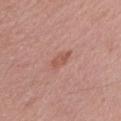Q: Is there a histopathology result?
A: no biopsy performed (imaged during a skin exam)
Q: Patient demographics?
A: female, roughly 50 years of age
Q: What kind of image is this?
A: ~15 mm crop, total-body skin-cancer survey
Q: Illumination type?
A: white-light illumination
Q: What did automated image analysis measure?
A: roughly 8 lightness units darker than nearby skin; border irregularity of about 3 on a 0–10 scale; a classifier nevus-likeness of about 10/100 and a lesion-detection confidence of about 100/100
Q: How large is the lesion?
A: about 3 mm
Q: Lesion location?
A: the left upper arm